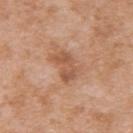  biopsy_status: not biopsied; imaged during a skin examination
  image:
    source: total-body photography crop
    field_of_view_mm: 15
  site: upper back
  lighting: white-light
  lesion_size:
    long_diameter_mm_approx: 3.5
  patient:
    sex: female
    age_approx: 40